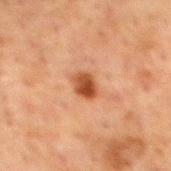| field | value |
|---|---|
| biopsy status | no biopsy performed (imaged during a skin exam) |
| lesion size | ~2.5 mm (longest diameter) |
| subject | male, aged 58 to 62 |
| imaging modality | ~15 mm crop, total-body skin-cancer survey |
| illumination | cross-polarized illumination |
| location | the back |